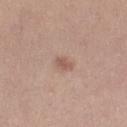The lesion was photographed on a routine skin check and not biopsied; there is no pathology result.
Approximately 2.5 mm at its widest.
An algorithmic analysis of the crop reported a nevus-likeness score of about 10/100 and a lesion-detection confidence of about 100/100.
The lesion is located on the leg.
This is a white-light tile.
A female patient, about 25 years old.
A 15 mm close-up extracted from a 3D total-body photography capture.